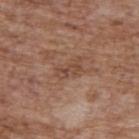diameter: about 3.5 mm; image source: 15 mm crop, total-body photography; location: the upper back; patient: male, about 70 years old.